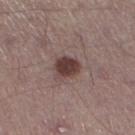Q: Was this lesion biopsied?
A: catalogued during a skin exam; not biopsied
Q: Who is the patient?
A: male, in their mid- to late 40s
Q: Lesion size?
A: about 3 mm
Q: Where on the body is the lesion?
A: the left lower leg
Q: What is the imaging modality?
A: 15 mm crop, total-body photography
Q: Illumination type?
A: white-light illumination
Q: What did automated image analysis measure?
A: an area of roughly 5.5 mm², an outline eccentricity of about 0.6 (0 = round, 1 = elongated), and a shape-asymmetry score of about 0.2 (0 = symmetric); a lesion color around L≈38 a*≈18 b*≈19 in CIELAB, about 14 CIELAB-L* units darker than the surrounding skin, and a normalized border contrast of about 11.5